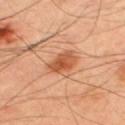Clinical impression:
The lesion was photographed on a routine skin check and not biopsied; there is no pathology result.
Acquisition and patient details:
Located on the upper back. Measured at roughly 3.5 mm in maximum diameter. A male subject, aged 48 to 52. Captured under cross-polarized illumination. This image is a 15 mm lesion crop taken from a total-body photograph.